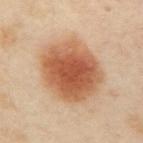This lesion was catalogued during total-body skin photography and was not selected for biopsy. A region of skin cropped from a whole-body photographic capture, roughly 15 mm wide. The patient is a female in their 40s. The lesion is on the arm.